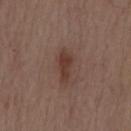Q: Is there a histopathology result?
A: imaged on a skin check; not biopsied
Q: Patient demographics?
A: male, aged approximately 70
Q: What lighting was used for the tile?
A: white-light illumination
Q: Lesion size?
A: ≈3.5 mm
Q: Where on the body is the lesion?
A: the back
Q: What did automated image analysis measure?
A: a lesion color around L≈37 a*≈19 b*≈24 in CIELAB, a lesion–skin lightness drop of about 9, and a normalized border contrast of about 8; a border-irregularity rating of about 3.5/10, a within-lesion color-variation index near 1.5/10, and radial color variation of about 0.5
Q: What is the imaging modality?
A: ~15 mm tile from a whole-body skin photo